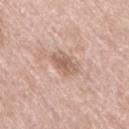Clinical impression: The lesion was tiled from a total-body skin photograph and was not biopsied. Background: The tile uses white-light illumination. A female subject approximately 60 years of age. This image is a 15 mm lesion crop taken from a total-body photograph. Measured at roughly 3.5 mm in maximum diameter. On the right thigh. The lesion-visualizer software estimated an area of roughly 5.5 mm² and an eccentricity of roughly 0.8. The software also gave a mean CIELAB color near L≈60 a*≈19 b*≈28, roughly 10 lightness units darker than nearby skin, and a normalized lesion–skin contrast near 6.5. And it measured a border-irregularity rating of about 3/10.